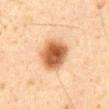Clinical impression: The lesion was tiled from a total-body skin photograph and was not biopsied. Clinical summary: Approximately 4.5 mm at its widest. On the abdomen. The patient is a male aged approximately 60. A 15 mm close-up tile from a total-body photography series done for melanoma screening. Automated tile analysis of the lesion measured a border-irregularity rating of about 1.5/10, a color-variation rating of about 6/10, and radial color variation of about 1.5. The analysis additionally found an automated nevus-likeness rating near 100 out of 100 and a detector confidence of about 100 out of 100 that the crop contains a lesion. The tile uses cross-polarized illumination.